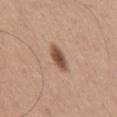This lesion was catalogued during total-body skin photography and was not selected for biopsy.
Cropped from a total-body skin-imaging series; the visible field is about 15 mm.
Located on the mid back.
A male patient aged around 65.
The lesion's longest dimension is about 3.5 mm.
This is a white-light tile.